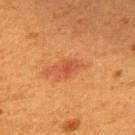The lesion was photographed on a routine skin check and not biopsied; there is no pathology result. From the upper back. A lesion tile, about 15 mm wide, cut from a 3D total-body photograph. Automated tile analysis of the lesion measured a lesion color around L≈44 a*≈27 b*≈34 in CIELAB, a lesion–skin lightness drop of about 7, and a normalized lesion–skin contrast near 5.5. It also reported a color-variation rating of about 2/10 and peripheral color asymmetry of about 0.5. The subject is a female about 50 years old. This is a cross-polarized tile.